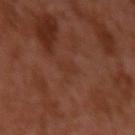Q: Was this lesion biopsied?
A: total-body-photography surveillance lesion; no biopsy
Q: What is the anatomic site?
A: the left upper arm
Q: What are the patient's age and sex?
A: male, aged 28 to 32
Q: How large is the lesion?
A: ≈2.5 mm
Q: How was this image acquired?
A: 15 mm crop, total-body photography